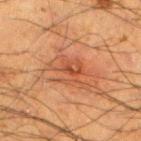Imaged during a routine full-body skin examination; the lesion was not biopsied and no histopathology is available.
Automated image analysis of the tile measured a lesion color around L≈40 a*≈23 b*≈31 in CIELAB, about 7 CIELAB-L* units darker than the surrounding skin, and a normalized lesion–skin contrast near 6.
A lesion tile, about 15 mm wide, cut from a 3D total-body photograph.
About 5 mm across.
On the leg.
A male subject, roughly 35 years of age.